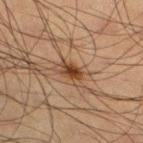Notes:
- follow-up: no biopsy performed (imaged during a skin exam)
- patient: male, aged approximately 35
- image source: ~15 mm tile from a whole-body skin photo
- site: the left lower leg